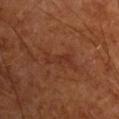No biopsy was performed on this lesion — it was imaged during a full skin examination and was not determined to be concerning.
Approximately 4 mm at its widest.
Located on the right upper arm.
A close-up tile cropped from a whole-body skin photograph, about 15 mm across.
A male subject, aged around 65.
The tile uses cross-polarized illumination.
The total-body-photography lesion software estimated a lesion area of about 4.5 mm², an eccentricity of roughly 0.95, and a symmetry-axis asymmetry near 0.3. And it measured an average lesion color of about L≈31 a*≈24 b*≈28 (CIELAB) and a normalized border contrast of about 5.5. The software also gave a border-irregularity index near 6/10 and internal color variation of about 0.5 on a 0–10 scale.